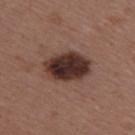Captured during whole-body skin photography for melanoma surveillance; the lesion was not biopsied. A lesion tile, about 15 mm wide, cut from a 3D total-body photograph. Imaged with white-light lighting. A female subject approximately 45 years of age. The lesion is on the right upper arm. Automated image analysis of the tile measured lesion-presence confidence of about 100/100.